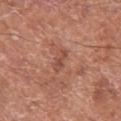Captured during whole-body skin photography for melanoma surveillance; the lesion was not biopsied. The lesion is located on the left thigh. Captured under white-light illumination. Longest diameter approximately 3 mm. A male subject in their mid- to late 60s. This image is a 15 mm lesion crop taken from a total-body photograph. An algorithmic analysis of the crop reported a footprint of about 4 mm² and an eccentricity of roughly 0.85. And it measured a lesion–skin lightness drop of about 7 and a lesion-to-skin contrast of about 5.5 (normalized; higher = more distinct). The software also gave peripheral color asymmetry of about 1. It also reported a nevus-likeness score of about 0/100 and a lesion-detection confidence of about 100/100.